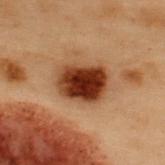follow-up: total-body-photography surveillance lesion; no biopsy
image source: ~15 mm crop, total-body skin-cancer survey
lesion diameter: about 5 mm
anatomic site: the upper back
image-analysis metrics: a lesion color around L≈31 a*≈21 b*≈29 in CIELAB, about 17 CIELAB-L* units darker than the surrounding skin, and a lesion-to-skin contrast of about 14.5 (normalized; higher = more distinct); border irregularity of about 1.5 on a 0–10 scale, internal color variation of about 7.5 on a 0–10 scale, and radial color variation of about 2
subject: male, aged 53–57
tile lighting: cross-polarized